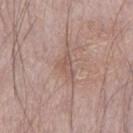Q: What is the lesion's diameter?
A: about 3.5 mm
Q: Lesion location?
A: the left thigh
Q: How was the tile lit?
A: white-light illumination
Q: Automated lesion metrics?
A: a lesion color around L≈55 a*≈18 b*≈24 in CIELAB, about 7 CIELAB-L* units darker than the surrounding skin, and a normalized border contrast of about 5.5; a nevus-likeness score of about 0/100 and lesion-presence confidence of about 70/100
Q: Who is the patient?
A: male, about 65 years old
Q: What kind of image is this?
A: ~15 mm tile from a whole-body skin photo